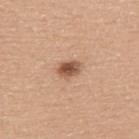Q: Was a biopsy performed?
A: imaged on a skin check; not biopsied
Q: What is the imaging modality?
A: ~15 mm crop, total-body skin-cancer survey
Q: Automated lesion metrics?
A: an area of roughly 5 mm², an eccentricity of roughly 0.75, and a shape-asymmetry score of about 0.2 (0 = symmetric); a lesion–skin lightness drop of about 14 and a lesion-to-skin contrast of about 9.5 (normalized; higher = more distinct); a nevus-likeness score of about 95/100 and lesion-presence confidence of about 100/100
Q: What are the patient's age and sex?
A: male, aged around 40
Q: How large is the lesion?
A: ~3 mm (longest diameter)
Q: What is the anatomic site?
A: the upper back